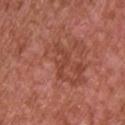Q: What lighting was used for the tile?
A: white-light
Q: Who is the patient?
A: male, aged around 65
Q: Where on the body is the lesion?
A: the upper back
Q: What is the imaging modality?
A: ~15 mm tile from a whole-body skin photo
Q: How large is the lesion?
A: about 3.5 mm